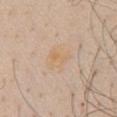Impression: No biopsy was performed on this lesion — it was imaged during a full skin examination and was not determined to be concerning. Image and clinical context: Cropped from a total-body skin-imaging series; the visible field is about 15 mm. The recorded lesion diameter is about 2.5 mm. The tile uses white-light illumination. The lesion-visualizer software estimated a lesion area of about 3.5 mm² and an outline eccentricity of about 0.7 (0 = round, 1 = elongated). The analysis additionally found a mean CIELAB color near L≈66 a*≈16 b*≈36, roughly 4 lightness units darker than nearby skin, and a normalized lesion–skin contrast near 5.5. It also reported a border-irregularity index near 4/10, internal color variation of about 3 on a 0–10 scale, and peripheral color asymmetry of about 1. On the chest. A male patient about 60 years old.